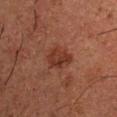The lesion was tiled from a total-body skin photograph and was not biopsied.
A male patient aged 48–52.
A region of skin cropped from a whole-body photographic capture, roughly 15 mm wide.
Located on the right upper arm.